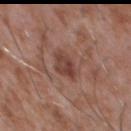| field | value |
|---|---|
| notes | imaged on a skin check; not biopsied |
| image | total-body-photography crop, ~15 mm field of view |
| anatomic site | the chest |
| patient | male, aged 43–47 |